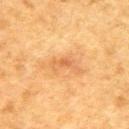Clinical impression: The lesion was tiled from a total-body skin photograph and was not biopsied. Context: The lesion is located on the back. The lesion's longest dimension is about 4 mm. A close-up tile cropped from a whole-body skin photograph, about 15 mm across. A male patient aged 48 to 52. Captured under cross-polarized illumination.